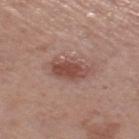Clinical impression:
Imaged during a routine full-body skin examination; the lesion was not biopsied and no histopathology is available.
Background:
A female subject roughly 55 years of age. Captured under white-light illumination. About 4.5 mm across. The lesion is located on the right thigh. This image is a 15 mm lesion crop taken from a total-body photograph.